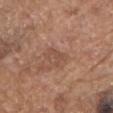workup — imaged on a skin check; not biopsied | site — the head or neck | patient — male, approximately 80 years of age | image source — ~15 mm crop, total-body skin-cancer survey.